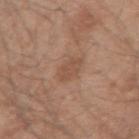notes=catalogued during a skin exam; not biopsied
lesion size=≈3 mm
subject=male, aged 43–47
tile lighting=white-light
body site=the right upper arm
image=total-body-photography crop, ~15 mm field of view